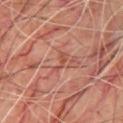This lesion was catalogued during total-body skin photography and was not selected for biopsy. A close-up tile cropped from a whole-body skin photograph, about 15 mm across. Located on the chest. Imaged with cross-polarized lighting. The lesion's longest dimension is about 3 mm. A male patient aged around 70.